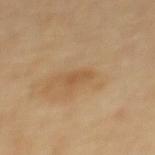| field | value |
|---|---|
| notes | catalogued during a skin exam; not biopsied |
| subject | female, in their 70s |
| site | the upper back |
| imaging modality | total-body-photography crop, ~15 mm field of view |